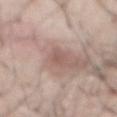The lesion was tiled from a total-body skin photograph and was not biopsied.
The lesion is located on the abdomen.
The tile uses white-light illumination.
The recorded lesion diameter is about 3 mm.
A male subject in their mid- to late 50s.
A 15 mm close-up tile from a total-body photography series done for melanoma screening.